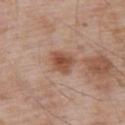This lesion was catalogued during total-body skin photography and was not selected for biopsy. Located on the upper back. A male subject in their mid- to late 50s. A close-up tile cropped from a whole-body skin photograph, about 15 mm across.